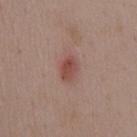The lesion was tiled from a total-body skin photograph and was not biopsied.
This is a white-light tile.
A 15 mm crop from a total-body photograph taken for skin-cancer surveillance.
A female subject approximately 35 years of age.
The total-body-photography lesion software estimated a shape eccentricity near 0.7 and a shape-asymmetry score of about 0.25 (0 = symmetric). It also reported a color-variation rating of about 3/10 and peripheral color asymmetry of about 1. The analysis additionally found an automated nevus-likeness rating near 95 out of 100.
The lesion is on the chest.